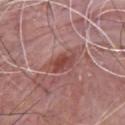{
  "biopsy_status": "not biopsied; imaged during a skin examination",
  "lesion_size": {
    "long_diameter_mm_approx": 4.0
  },
  "patient": {
    "sex": "male",
    "age_approx": 70
  },
  "lighting": "white-light",
  "site": "chest",
  "image": {
    "source": "total-body photography crop",
    "field_of_view_mm": 15
  }
}